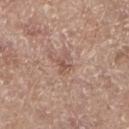biopsy_status: not biopsied; imaged during a skin examination
patient:
  sex: female
  age_approx: 75
site: left lower leg
automated_metrics:
  area_mm2_approx: 3.0
  eccentricity: 0.7
  shape_asymmetry: 0.4
  color_variation_0_10: 0.5
  peripheral_color_asymmetry: 0.0
lesion_size:
  long_diameter_mm_approx: 2.5
image:
  source: total-body photography crop
  field_of_view_mm: 15